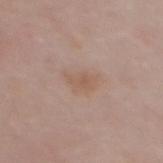No biopsy was performed on this lesion — it was imaged during a full skin examination and was not determined to be concerning. A 15 mm crop from a total-body photograph taken for skin-cancer surveillance. A female patient roughly 50 years of age. The lesion is on the mid back. Automated image analysis of the tile measured a lesion area of about 5.5 mm² and an eccentricity of roughly 0.8. The software also gave a mean CIELAB color near L≈57 a*≈17 b*≈27, a lesion–skin lightness drop of about 6, and a lesion-to-skin contrast of about 5.5 (normalized; higher = more distinct). It also reported internal color variation of about 2 on a 0–10 scale. This is a white-light tile. About 3.5 mm across.